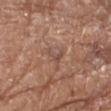<record>
<biopsy_status>not biopsied; imaged during a skin examination</biopsy_status>
<site>right thigh</site>
<patient>
  <sex>female</sex>
  <age_approx>75</age_approx>
</patient>
<lighting>white-light</lighting>
<lesion_size>
  <long_diameter_mm_approx>3.0</long_diameter_mm_approx>
</lesion_size>
<image>
  <source>total-body photography crop</source>
  <field_of_view_mm>15</field_of_view_mm>
</image>
</record>